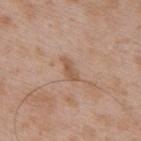The lesion was tiled from a total-body skin photograph and was not biopsied. Located on the mid back. A male patient, in their 50s. A 15 mm close-up extracted from a 3D total-body photography capture. Measured at roughly 3 mm in maximum diameter. Automated tile analysis of the lesion measured a footprint of about 3 mm². The software also gave a nevus-likeness score of about 0/100 and a detector confidence of about 100 out of 100 that the crop contains a lesion. The tile uses white-light illumination.